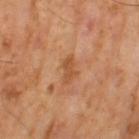No biopsy was performed on this lesion — it was imaged during a full skin examination and was not determined to be concerning. A male patient, aged approximately 65. Cropped from a whole-body photographic skin survey; the tile spans about 15 mm. Imaged with cross-polarized lighting. Longest diameter approximately 3 mm. Automated image analysis of the tile measured border irregularity of about 4 on a 0–10 scale and a color-variation rating of about 1/10.